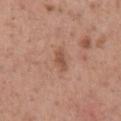Notes:
– subject: male, aged 43 to 47
– automated metrics: a lesion area of about 3 mm², a shape eccentricity near 0.85, and a shape-asymmetry score of about 0.35 (0 = symmetric); a lesion color around L≈52 a*≈22 b*≈30 in CIELAB; border irregularity of about 3 on a 0–10 scale, a color-variation rating of about 1/10, and peripheral color asymmetry of about 0.5
– tile lighting: white-light illumination
– diameter: about 2.5 mm
– body site: the chest
– image source: ~15 mm tile from a whole-body skin photo